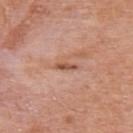Q: Was a biopsy performed?
A: no biopsy performed (imaged during a skin exam)
Q: Lesion location?
A: the upper back
Q: Patient demographics?
A: male, aged around 75
Q: What did automated image analysis measure?
A: a mean CIELAB color near L≈54 a*≈24 b*≈31, about 11 CIELAB-L* units darker than the surrounding skin, and a normalized border contrast of about 7.5; a nevus-likeness score of about 0/100 and a detector confidence of about 95 out of 100 that the crop contains a lesion
Q: What kind of image is this?
A: 15 mm crop, total-body photography
Q: How was the tile lit?
A: white-light illumination
Q: What is the lesion's diameter?
A: about 2.5 mm